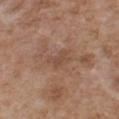Recorded during total-body skin imaging; not selected for excision or biopsy. The lesion is on the chest. A 15 mm close-up extracted from a 3D total-body photography capture. A male subject, aged 73 to 77.